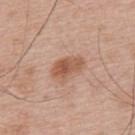Recorded during total-body skin imaging; not selected for excision or biopsy. The lesion-visualizer software estimated a lesion area of about 7.5 mm², an eccentricity of roughly 0.8, and a shape-asymmetry score of about 0.2 (0 = symmetric). And it measured a lesion color around L≈55 a*≈22 b*≈31 in CIELAB, about 11 CIELAB-L* units darker than the surrounding skin, and a normalized border contrast of about 8. The software also gave a border-irregularity rating of about 2.5/10, a color-variation rating of about 4/10, and peripheral color asymmetry of about 1. And it measured an automated nevus-likeness rating near 70 out of 100 and a lesion-detection confidence of about 100/100. A lesion tile, about 15 mm wide, cut from a 3D total-body photograph. A male subject aged approximately 65. From the upper back. The recorded lesion diameter is about 4 mm. Captured under white-light illumination.